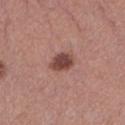biopsy status = total-body-photography surveillance lesion; no biopsy | diameter = ≈3 mm | acquisition = ~15 mm crop, total-body skin-cancer survey | subject = female, in their 60s | illumination = white-light illumination | anatomic site = the left lower leg.